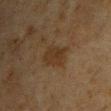workup: no biopsy performed (imaged during a skin exam) | body site: the chest | image: 15 mm crop, total-body photography | diameter: ~4 mm (longest diameter) | subject: male, approximately 45 years of age | tile lighting: cross-polarized | image-analysis metrics: a lesion area of about 8.5 mm², an outline eccentricity of about 0.8 (0 = round, 1 = elongated), and a shape-asymmetry score of about 0.2 (0 = symmetric); a lesion color around L≈27 a*≈13 b*≈25 in CIELAB, about 5 CIELAB-L* units darker than the surrounding skin, and a lesion-to-skin contrast of about 6.5 (normalized; higher = more distinct); border irregularity of about 3 on a 0–10 scale, a within-lesion color-variation index near 3/10, and a peripheral color-asymmetry measure near 1; an automated nevus-likeness rating near 10 out of 100 and a lesion-detection confidence of about 100/100.